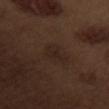Case summary:
– subject: male, aged 68 to 72
– lighting: white-light illumination
– size: ~3.5 mm (longest diameter)
– site: the arm
– image: ~15 mm tile from a whole-body skin photo